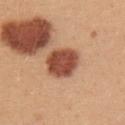| field | value |
|---|---|
| notes | imaged on a skin check; not biopsied |
| automated metrics | a footprint of about 13 mm²; a mean CIELAB color near L≈50 a*≈26 b*≈32, a lesion–skin lightness drop of about 17, and a lesion-to-skin contrast of about 11.5 (normalized; higher = more distinct); a border-irregularity rating of about 1.5/10, a color-variation rating of about 3/10, and peripheral color asymmetry of about 1 |
| lighting | white-light illumination |
| subject | female, in their mid-20s |
| site | the upper back |
| image | 15 mm crop, total-body photography |
| diameter | ≈4 mm |